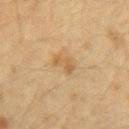Part of a total-body skin-imaging series; this lesion was reviewed on a skin check and was not flagged for biopsy. A female patient in their 40s. This is a cross-polarized tile. Cropped from a total-body skin-imaging series; the visible field is about 15 mm. On the right upper arm. The lesion's longest dimension is about 3.5 mm.